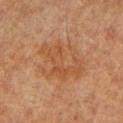Captured during whole-body skin photography for melanoma surveillance; the lesion was not biopsied. A male subject in their mid-60s. The tile uses cross-polarized illumination. Cropped from a whole-body photographic skin survey; the tile spans about 15 mm. From the right forearm. Measured at roughly 6 mm in maximum diameter.